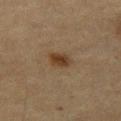workup — total-body-photography surveillance lesion; no biopsy | image — ~15 mm crop, total-body skin-cancer survey | location — the left thigh | patient — female, in their mid- to late 50s | size — about 3 mm | tile lighting — cross-polarized.